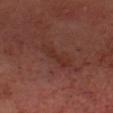| feature | finding |
|---|---|
| workup | no biopsy performed (imaged during a skin exam) |
| lighting | cross-polarized |
| image-analysis metrics | a lesion color around L≈31 a*≈23 b*≈25 in CIELAB, roughly 5 lightness units darker than nearby skin, and a normalized lesion–skin contrast near 5.5 |
| anatomic site | the head or neck |
| imaging modality | 15 mm crop, total-body photography |
| patient | male, in their mid- to late 50s |
| lesion size | ~4 mm (longest diameter) |